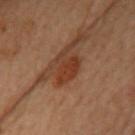Background:
A region of skin cropped from a whole-body photographic capture, roughly 15 mm wide. Imaged with cross-polarized lighting. The total-body-photography lesion software estimated a border-irregularity index near 4.5/10, a color-variation rating of about 1.5/10, and radial color variation of about 0.5. A female subject, roughly 60 years of age. The recorded lesion diameter is about 4 mm. The lesion is located on the chest.